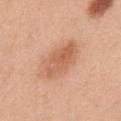  biopsy_status: not biopsied; imaged during a skin examination
  lesion_size:
    long_diameter_mm_approx: 6.0
  automated_metrics:
    area_mm2_approx: 14.0
    eccentricity: 0.85
    shape_asymmetry: 0.15
    nevus_likeness_0_100: 95
    lesion_detection_confidence_0_100: 100
  patient:
    sex: male
    age_approx: 50
  lighting: white-light
  site: chest
  image:
    source: total-body photography crop
    field_of_view_mm: 15